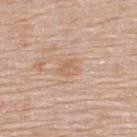Clinical impression: Imaged during a routine full-body skin examination; the lesion was not biopsied and no histopathology is available. Acquisition and patient details: Measured at roughly 2.5 mm in maximum diameter. Captured under white-light illumination. A lesion tile, about 15 mm wide, cut from a 3D total-body photograph. The lesion is located on the upper back. Automated tile analysis of the lesion measured an area of roughly 4 mm², an outline eccentricity of about 0.65 (0 = round, 1 = elongated), and two-axis asymmetry of about 0.3. The software also gave roughly 6 lightness units darker than nearby skin and a normalized border contrast of about 5. The subject is a male roughly 80 years of age.